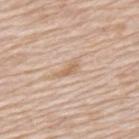{"biopsy_status": "not biopsied; imaged during a skin examination", "patient": {"sex": "male", "age_approx": 80}, "site": "back", "lesion_size": {"long_diameter_mm_approx": 2.5}, "lighting": "white-light", "image": {"source": "total-body photography crop", "field_of_view_mm": 15}}